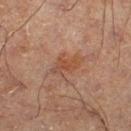notes: no biopsy performed (imaged during a skin exam) | automated lesion analysis: a lesion area of about 4 mm² and a shape-asymmetry score of about 0.35 (0 = symmetric); a mean CIELAB color near L≈41 a*≈20 b*≈28, about 6 CIELAB-L* units darker than the surrounding skin, and a normalized border contrast of about 6; a classifier nevus-likeness of about 20/100 | acquisition: 15 mm crop, total-body photography | patient: male, aged approximately 65 | site: the right lower leg | lighting: cross-polarized illumination.